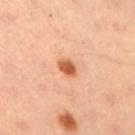* notes — no biopsy performed (imaged during a skin exam)
* subject — female, aged around 35
* anatomic site — the right upper arm
* image — ~15 mm tile from a whole-body skin photo
* tile lighting — cross-polarized illumination